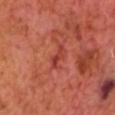{
  "patient": {
    "sex": "male",
    "age_approx": 65
  },
  "image": {
    "source": "total-body photography crop",
    "field_of_view_mm": 15
  }
}